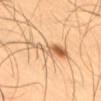Q: Is there a histopathology result?
A: imaged on a skin check; not biopsied
Q: Patient demographics?
A: male, aged approximately 55
Q: Lesion location?
A: the right thigh
Q: What lighting was used for the tile?
A: cross-polarized
Q: What is the imaging modality?
A: ~15 mm crop, total-body skin-cancer survey
Q: Automated lesion metrics?
A: border irregularity of about 6.5 on a 0–10 scale, a color-variation rating of about 8.5/10, and peripheral color asymmetry of about 2; an automated nevus-likeness rating near 100 out of 100
Q: What is the lesion's diameter?
A: ≈5.5 mm